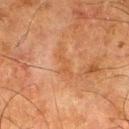Impression:
The lesion was tiled from a total-body skin photograph and was not biopsied.
Clinical summary:
From the right thigh. A roughly 15 mm field-of-view crop from a total-body skin photograph. A male subject about 80 years old.